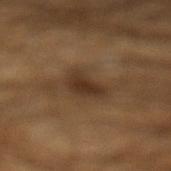workup = total-body-photography surveillance lesion; no biopsy | location = the left lower leg | patient = male, aged 43 to 47 | diameter = ~3.5 mm (longest diameter) | image source = total-body-photography crop, ~15 mm field of view | lighting = cross-polarized.